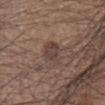Clinical impression: No biopsy was performed on this lesion — it was imaged during a full skin examination and was not determined to be concerning. Background: The total-body-photography lesion software estimated a border-irregularity index near 2.5/10 and a peripheral color-asymmetry measure near 1. The analysis additionally found a nevus-likeness score of about 10/100 and a lesion-detection confidence of about 100/100. Approximately 3.5 mm at its widest. This is a white-light tile. The subject is a male aged around 45. A 15 mm close-up tile from a total-body photography series done for melanoma screening. From the right lower leg.